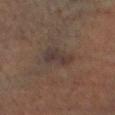Image and clinical context: From the right lower leg. A male patient, approximately 70 years of age. This image is a 15 mm lesion crop taken from a total-body photograph.